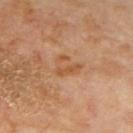Imaged during a routine full-body skin examination; the lesion was not biopsied and no histopathology is available. A 15 mm close-up extracted from a 3D total-body photography capture. The tile uses cross-polarized illumination. About 3 mm across. Automated tile analysis of the lesion measured a mean CIELAB color near L≈53 a*≈22 b*≈36, about 7 CIELAB-L* units darker than the surrounding skin, and a lesion-to-skin contrast of about 6 (normalized; higher = more distinct). The software also gave an automated nevus-likeness rating near 0 out of 100 and lesion-presence confidence of about 100/100. The subject is a male approximately 70 years of age. On the mid back.